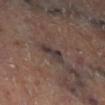Q: Was a biopsy performed?
A: no biopsy performed (imaged during a skin exam)
Q: Lesion location?
A: the right lower leg
Q: What did automated image analysis measure?
A: an automated nevus-likeness rating near 0 out of 100 and a lesion-detection confidence of about 55/100
Q: How was the tile lit?
A: cross-polarized illumination
Q: What kind of image is this?
A: total-body-photography crop, ~15 mm field of view